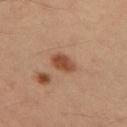• notes: catalogued during a skin exam; not biopsied
• image-analysis metrics: an area of roughly 5.5 mm² and an eccentricity of roughly 0.75; border irregularity of about 1.5 on a 0–10 scale and peripheral color asymmetry of about 1; a classifier nevus-likeness of about 90/100 and a detector confidence of about 100 out of 100 that the crop contains a lesion
• size: about 3 mm
• subject: male, roughly 40 years of age
• body site: the leg
• tile lighting: cross-polarized illumination
• image: 15 mm crop, total-body photography